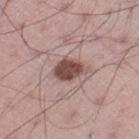biopsy_status: not biopsied; imaged during a skin examination
lighting: white-light
automated_metrics:
  cielab_L: 48
  cielab_a: 20
  cielab_b: 22
  vs_skin_darker_L: 15.0
  vs_skin_contrast_norm: 11.0
  border_irregularity_0_10: 2.0
  peripheral_color_asymmetry: 1.0
  nevus_likeness_0_100: 95
  lesion_detection_confidence_0_100: 100
patient:
  sex: male
  age_approx: 45
site: leg
image:
  source: total-body photography crop
  field_of_view_mm: 15